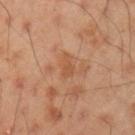This lesion was catalogued during total-body skin photography and was not selected for biopsy. From the left upper arm. A region of skin cropped from a whole-body photographic capture, roughly 15 mm wide. Automated tile analysis of the lesion measured a lesion area of about 3 mm², an outline eccentricity of about 0.9 (0 = round, 1 = elongated), and two-axis asymmetry of about 0.4. A male patient, aged 43 to 47. Captured under cross-polarized illumination.